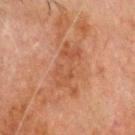Notes:
– notes · imaged on a skin check; not biopsied
– subject · male, approximately 80 years of age
– illumination · cross-polarized
– lesion diameter · about 5.5 mm
– body site · the head or neck
– acquisition · total-body-photography crop, ~15 mm field of view